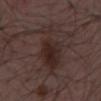Assessment: Imaged during a routine full-body skin examination; the lesion was not biopsied and no histopathology is available. Background: The lesion is located on the front of the torso. A 15 mm close-up extracted from a 3D total-body photography capture. The patient is a male roughly 55 years of age. The recorded lesion diameter is about 7.5 mm. This is a white-light tile.